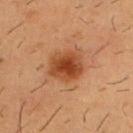<tbp_lesion>
<biopsy_status>not biopsied; imaged during a skin examination</biopsy_status>
<patient>
  <sex>male</sex>
  <age_approx>55</age_approx>
</patient>
<image>
  <source>total-body photography crop</source>
  <field_of_view_mm>15</field_of_view_mm>
</image>
<lesion_size>
  <long_diameter_mm_approx>4.0</long_diameter_mm_approx>
</lesion_size>
<lighting>cross-polarized</lighting>
<site>chest</site>
</tbp_lesion>